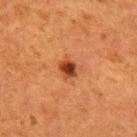Captured during whole-body skin photography for melanoma surveillance; the lesion was not biopsied.
From the arm.
A region of skin cropped from a whole-body photographic capture, roughly 15 mm wide.
The subject is a female aged 48–52.
Longest diameter approximately 2.5 mm.